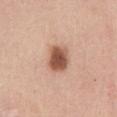Case summary:
• follow-up: total-body-photography surveillance lesion; no biopsy
• illumination: white-light illumination
• image source: ~15 mm tile from a whole-body skin photo
• TBP lesion metrics: a border-irregularity rating of about 1.5/10; an automated nevus-likeness rating near 100 out of 100 and lesion-presence confidence of about 100/100
• site: the abdomen
• patient: female, aged approximately 35